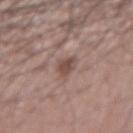  biopsy_status: not biopsied; imaged during a skin examination
  automated_metrics:
    cielab_L: 48
    cielab_a: 18
    cielab_b: 22
    vs_skin_darker_L: 10.0
    vs_skin_contrast_norm: 7.5
    border_irregularity_0_10: 2.5
    color_variation_0_10: 2.0
    peripheral_color_asymmetry: 0.5
  patient:
    sex: male
    age_approx: 50
  image:
    source: total-body photography crop
    field_of_view_mm: 15
  site: right forearm
  lighting: white-light
  lesion_size:
    long_diameter_mm_approx: 3.0